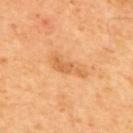The lesion was photographed on a routine skin check and not biopsied; there is no pathology result. This image is a 15 mm lesion crop taken from a total-body photograph. A male patient, in their mid- to late 60s. Approximately 4.5 mm at its widest. Captured under cross-polarized illumination. Located on the upper back. Automated image analysis of the tile measured an average lesion color of about L≈61 a*≈25 b*≈43 (CIELAB), a lesion–skin lightness drop of about 9, and a normalized border contrast of about 6. The software also gave a within-lesion color-variation index near 1/10 and radial color variation of about 0. And it measured a classifier nevus-likeness of about 5/100 and lesion-presence confidence of about 100/100.